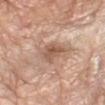Cropped from a whole-body photographic skin survey; the tile spans about 15 mm. The lesion is on the left forearm. The subject is a female aged approximately 75.